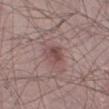Imaged during a routine full-body skin examination; the lesion was not biopsied and no histopathology is available. A male patient aged approximately 25. The lesion is on the right lower leg. The total-body-photography lesion software estimated a classifier nevus-likeness of about 70/100 and a detector confidence of about 100 out of 100 that the crop contains a lesion. About 3 mm across. Captured under white-light illumination. A roughly 15 mm field-of-view crop from a total-body skin photograph.